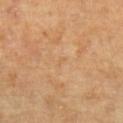Recorded during total-body skin imaging; not selected for excision or biopsy.
Imaged with cross-polarized lighting.
On the left forearm.
A region of skin cropped from a whole-body photographic capture, roughly 15 mm wide.
Approximately 1.5 mm at its widest.
The patient is a female in their mid- to late 60s.